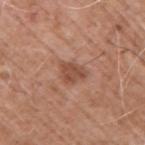Impression: Recorded during total-body skin imaging; not selected for excision or biopsy. Clinical summary: The lesion-visualizer software estimated an automated nevus-likeness rating near 20 out of 100 and a detector confidence of about 100 out of 100 that the crop contains a lesion. A male subject aged approximately 60. Measured at roughly 3.5 mm in maximum diameter. From the right upper arm. Imaged with white-light lighting. A close-up tile cropped from a whole-body skin photograph, about 15 mm across.